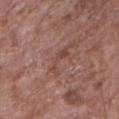Case summary:
* biopsy status: imaged on a skin check; not biopsied
* subject: female, about 70 years old
* body site: the right forearm
* image source: ~15 mm tile from a whole-body skin photo